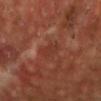Recorded during total-body skin imaging; not selected for excision or biopsy.
On the head or neck.
A male subject, in their mid- to late 50s.
A region of skin cropped from a whole-body photographic capture, roughly 15 mm wide.